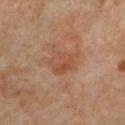biopsy status — imaged on a skin check; not biopsied
acquisition — ~15 mm tile from a whole-body skin photo
tile lighting — cross-polarized illumination
body site — the left lower leg
patient — female, about 50 years old
size — ≈3.5 mm